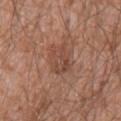Part of a total-body skin-imaging series; this lesion was reviewed on a skin check and was not flagged for biopsy. Measured at roughly 4 mm in maximum diameter. A region of skin cropped from a whole-body photographic capture, roughly 15 mm wide. From the lower back. A male subject, in their 60s. An algorithmic analysis of the crop reported a mean CIELAB color near L≈47 a*≈21 b*≈28, a lesion–skin lightness drop of about 7, and a normalized lesion–skin contrast near 5.5. The tile uses white-light illumination.